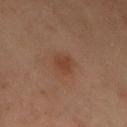The lesion was photographed on a routine skin check and not biopsied; there is no pathology result.
Located on the chest.
The lesion's longest dimension is about 2.5 mm.
A 15 mm close-up tile from a total-body photography series done for melanoma screening.
Imaged with cross-polarized lighting.
A female subject aged around 55.